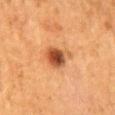Imaged during a routine full-body skin examination; the lesion was not biopsied and no histopathology is available.
About 3.5 mm across.
The patient is a female in their 50s.
Automated tile analysis of the lesion measured a footprint of about 8 mm², an eccentricity of roughly 0.5, and two-axis asymmetry of about 0.3. It also reported a lesion color around L≈43 a*≈23 b*≈34 in CIELAB and a normalized border contrast of about 10.
A region of skin cropped from a whole-body photographic capture, roughly 15 mm wide.
On the back.